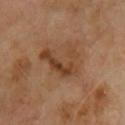Captured during whole-body skin photography for melanoma surveillance; the lesion was not biopsied. The recorded lesion diameter is about 5.5 mm. The lesion-visualizer software estimated an area of roughly 13 mm², a shape eccentricity near 0.75, and two-axis asymmetry of about 0.45. And it measured a color-variation rating of about 5/10 and a peripheral color-asymmetry measure near 1.5. A male patient roughly 65 years of age. Cropped from a total-body skin-imaging series; the visible field is about 15 mm. Captured under cross-polarized illumination. Located on the front of the torso.